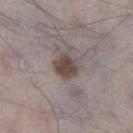No biopsy was performed on this lesion — it was imaged during a full skin examination and was not determined to be concerning. A 15 mm close-up tile from a total-body photography series done for melanoma screening. A male patient, aged around 70. Automated tile analysis of the lesion measured an average lesion color of about L≈44 a*≈13 b*≈19 (CIELAB), about 12 CIELAB-L* units darker than the surrounding skin, and a normalized border contrast of about 10. The software also gave a border-irregularity rating of about 2/10 and internal color variation of about 3 on a 0–10 scale. The tile uses white-light illumination. The lesion is located on the right lower leg. The lesion's longest dimension is about 3 mm.